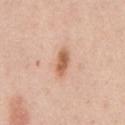Case summary:
– biopsy status: imaged on a skin check; not biopsied
– subject: male, about 60 years old
– anatomic site: the back
– acquisition: 15 mm crop, total-body photography